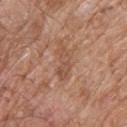Impression: Captured during whole-body skin photography for melanoma surveillance; the lesion was not biopsied. Background: A male subject, about 80 years old. The lesion-visualizer software estimated a lesion area of about 6 mm², an eccentricity of roughly 0.95, and two-axis asymmetry of about 0.35. It also reported an automated nevus-likeness rating near 0 out of 100 and a lesion-detection confidence of about 95/100. Located on the back. Cropped from a total-body skin-imaging series; the visible field is about 15 mm.